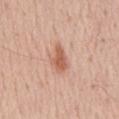No biopsy was performed on this lesion — it was imaged during a full skin examination and was not determined to be concerning. A 15 mm crop from a total-body photograph taken for skin-cancer surveillance. From the abdomen. About 3.5 mm across. A male patient, aged around 55.